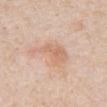The lesion was tiled from a total-body skin photograph and was not biopsied. This is a white-light tile. Measured at roughly 4.5 mm in maximum diameter. A male subject approximately 50 years of age. A lesion tile, about 15 mm wide, cut from a 3D total-body photograph. Located on the abdomen. An algorithmic analysis of the crop reported an area of roughly 9.5 mm², an eccentricity of roughly 0.75, and two-axis asymmetry of about 0.45. The software also gave an average lesion color of about L≈67 a*≈20 b*≈31 (CIELAB), about 8 CIELAB-L* units darker than the surrounding skin, and a lesion-to-skin contrast of about 5.5 (normalized; higher = more distinct). And it measured a border-irregularity index near 5.5/10 and radial color variation of about 1.